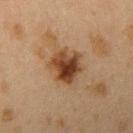Captured during whole-body skin photography for melanoma surveillance; the lesion was not biopsied.